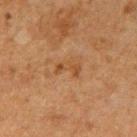lighting: cross-polarized illumination
size: ≈3 mm
site: the right upper arm
acquisition: ~15 mm tile from a whole-body skin photo
patient: male, aged approximately 50